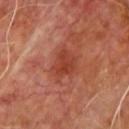| field | value |
|---|---|
| patient | male, aged around 60 |
| body site | the upper back |
| image | 15 mm crop, total-body photography |